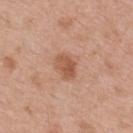Notes:
• follow-up · catalogued during a skin exam; not biopsied
• imaging modality · 15 mm crop, total-body photography
• illumination · white-light illumination
• automated lesion analysis · an area of roughly 5.5 mm², a shape eccentricity near 0.8, and a symmetry-axis asymmetry near 0.2; a mean CIELAB color near L≈55 a*≈23 b*≈32, a lesion–skin lightness drop of about 10, and a normalized lesion–skin contrast near 7.5; a border-irregularity index near 2/10, a color-variation rating of about 3.5/10, and radial color variation of about 1.5
• patient · male, aged 53–57
• anatomic site · the upper back
• size · ~3.5 mm (longest diameter)